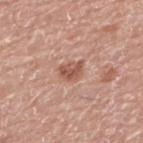Impression:
The lesion was tiled from a total-body skin photograph and was not biopsied.
Acquisition and patient details:
The recorded lesion diameter is about 3 mm. The total-body-photography lesion software estimated a border-irregularity index near 3/10, a color-variation rating of about 3/10, and radial color variation of about 1. And it measured a classifier nevus-likeness of about 85/100 and lesion-presence confidence of about 100/100. The tile uses white-light illumination. From the back. A male patient approximately 75 years of age. This image is a 15 mm lesion crop taken from a total-body photograph.